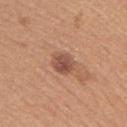Findings:
– follow-up · no biopsy performed (imaged during a skin exam)
– patient · female, approximately 45 years of age
– size · ≈2.5 mm
– body site · the upper back
– image source · ~15 mm tile from a whole-body skin photo
– image-analysis metrics · an eccentricity of roughly 0.45 and a shape-asymmetry score of about 0.15 (0 = symmetric); about 11 CIELAB-L* units darker than the surrounding skin and a lesion-to-skin contrast of about 8 (normalized; higher = more distinct)